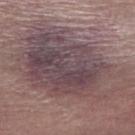workup — imaged on a skin check; not biopsied
subject — female, aged around 65
illumination — white-light illumination
site — the right forearm
automated lesion analysis — a lesion color around L≈45 a*≈16 b*≈14 in CIELAB and a normalized lesion–skin contrast near 9
imaging modality — 15 mm crop, total-body photography
lesion diameter — about 13.5 mm